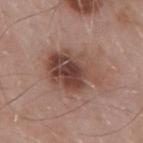Imaged during a routine full-body skin examination; the lesion was not biopsied and no histopathology is available.
The lesion is on the mid back.
A male patient aged 53 to 57.
A 15 mm close-up tile from a total-body photography series done for melanoma screening.